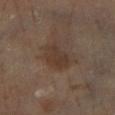This lesion was catalogued during total-body skin photography and was not selected for biopsy.
The tile uses cross-polarized illumination.
Cropped from a total-body skin-imaging series; the visible field is about 15 mm.
A male subject aged approximately 65.
The lesion-visualizer software estimated roughly 7 lightness units darker than nearby skin. And it measured a within-lesion color-variation index near 2/10 and peripheral color asymmetry of about 0.5. The software also gave lesion-presence confidence of about 100/100.
The lesion is located on the left leg.
Measured at roughly 4.5 mm in maximum diameter.